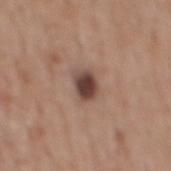biopsy_status: not biopsied; imaged during a skin examination
site: mid back
automated_metrics:
  area_mm2_approx: 6.0
  eccentricity: 0.7
  shape_asymmetry: 0.25
  nevus_likeness_0_100: 90
  lesion_detection_confidence_0_100: 100
lighting: white-light
patient:
  sex: male
  age_approx: 65
image:
  source: total-body photography crop
  field_of_view_mm: 15